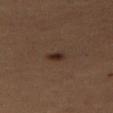Part of a total-body skin-imaging series; this lesion was reviewed on a skin check and was not flagged for biopsy. From the leg. The tile uses cross-polarized illumination. A 15 mm close-up tile from a total-body photography series done for melanoma screening. Approximately 2 mm at its widest. A female subject, in their mid-50s.